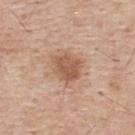Notes:
- workup: total-body-photography surveillance lesion; no biopsy
- image-analysis metrics: a border-irregularity index near 2/10 and peripheral color asymmetry of about 1; a lesion-detection confidence of about 100/100
- illumination: white-light
- anatomic site: the upper back
- patient: male, aged around 55
- image source: ~15 mm crop, total-body skin-cancer survey